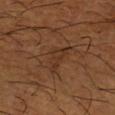Captured during whole-body skin photography for melanoma surveillance; the lesion was not biopsied.